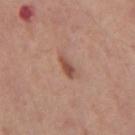Q: Was a biopsy performed?
A: catalogued during a skin exam; not biopsied
Q: What is the anatomic site?
A: the mid back
Q: How was the tile lit?
A: white-light
Q: What kind of image is this?
A: ~15 mm tile from a whole-body skin photo
Q: What did automated image analysis measure?
A: border irregularity of about 2.5 on a 0–10 scale, internal color variation of about 1.5 on a 0–10 scale, and a peripheral color-asymmetry measure near 0.5
Q: What is the lesion's diameter?
A: ~2.5 mm (longest diameter)
Q: What are the patient's age and sex?
A: male, in their 60s